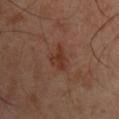follow-up: imaged on a skin check; not biopsied
tile lighting: cross-polarized illumination
subject: male, about 50 years old
location: the left arm
imaging modality: ~15 mm tile from a whole-body skin photo
size: ~3 mm (longest diameter)